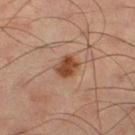The lesion was photographed on a routine skin check and not biopsied; there is no pathology result.
A 15 mm crop from a total-body photograph taken for skin-cancer surveillance.
Located on the right thigh.
The subject is a male in their 60s.
About 2.5 mm across.
Imaged with cross-polarized lighting.